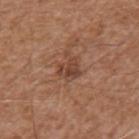This lesion was catalogued during total-body skin photography and was not selected for biopsy.
Automated tile analysis of the lesion measured border irregularity of about 3 on a 0–10 scale, a color-variation rating of about 3.5/10, and peripheral color asymmetry of about 1.5.
Longest diameter approximately 2.5 mm.
The tile uses white-light illumination.
Cropped from a total-body skin-imaging series; the visible field is about 15 mm.
A male patient, in their mid-70s.
The lesion is on the upper back.